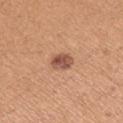No biopsy was performed on this lesion — it was imaged during a full skin examination and was not determined to be concerning.
A 15 mm close-up extracted from a 3D total-body photography capture.
The recorded lesion diameter is about 3 mm.
Captured under white-light illumination.
From the left upper arm.
A female subject aged 38 to 42.